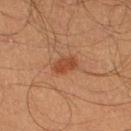<lesion>
<biopsy_status>not biopsied; imaged during a skin examination</biopsy_status>
</lesion>